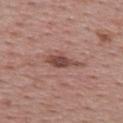{
  "biopsy_status": "not biopsied; imaged during a skin examination",
  "lighting": "white-light",
  "automated_metrics": {
    "color_variation_0_10": 2.5
  },
  "patient": {
    "sex": "female",
    "age_approx": 55
  },
  "image": {
    "source": "total-body photography crop",
    "field_of_view_mm": 15
  },
  "lesion_size": {
    "long_diameter_mm_approx": 4.5
  },
  "site": "upper back"
}